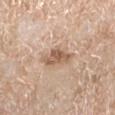| key | value |
|---|---|
| follow-up | no biopsy performed (imaged during a skin exam) |
| lesion diameter | ≈4.5 mm |
| site | the left lower leg |
| image | 15 mm crop, total-body photography |
| automated lesion analysis | a mean CIELAB color near L≈59 a*≈18 b*≈30 and roughly 12 lightness units darker than nearby skin; a border-irregularity rating of about 3/10, a within-lesion color-variation index near 4/10, and radial color variation of about 1.5; a nevus-likeness score of about 70/100 |
| subject | female, aged 68 to 72 |